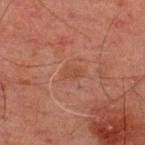A male patient about 45 years old. The lesion is on the upper back. A close-up tile cropped from a whole-body skin photograph, about 15 mm across. The total-body-photography lesion software estimated an outline eccentricity of about 0.8 (0 = round, 1 = elongated) and two-axis asymmetry of about 0.35. Captured under cross-polarized illumination.